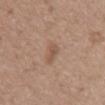biopsy status = catalogued during a skin exam; not biopsied | subject = female, aged 73–77 | anatomic site = the abdomen | acquisition = 15 mm crop, total-body photography.